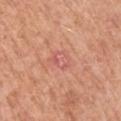Recorded during total-body skin imaging; not selected for excision or biopsy. A male subject roughly 50 years of age. A close-up tile cropped from a whole-body skin photograph, about 15 mm across. The lesion is on the left upper arm.